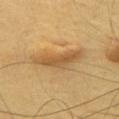Clinical impression: Captured during whole-body skin photography for melanoma surveillance; the lesion was not biopsied. Context: Located on the chest. A male patient approximately 65 years of age. This image is a 15 mm lesion crop taken from a total-body photograph.